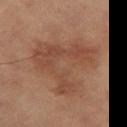Assessment:
Recorded during total-body skin imaging; not selected for excision or biopsy.
Clinical summary:
This is a cross-polarized tile. An algorithmic analysis of the crop reported a lesion area of about 32 mm², an outline eccentricity of about 0.25 (0 = round, 1 = elongated), and a symmetry-axis asymmetry near 0.55. The software also gave border irregularity of about 7.5 on a 0–10 scale, internal color variation of about 4 on a 0–10 scale, and a peripheral color-asymmetry measure near 1. A male patient, roughly 65 years of age. Longest diameter approximately 7.5 mm. A close-up tile cropped from a whole-body skin photograph, about 15 mm across. From the left thigh.